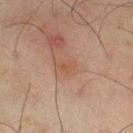Clinical impression: This lesion was catalogued during total-body skin photography and was not selected for biopsy. Context: Automated tile analysis of the lesion measured a footprint of about 3 mm², a shape eccentricity near 0.85, and a shape-asymmetry score of about 0.3 (0 = symmetric). The software also gave a lesion-to-skin contrast of about 5.5 (normalized; higher = more distinct). And it measured an automated nevus-likeness rating near 0 out of 100 and lesion-presence confidence of about 100/100. The patient is a male roughly 45 years of age. Located on the right thigh. The tile uses cross-polarized illumination. Approximately 2.5 mm at its widest. A roughly 15 mm field-of-view crop from a total-body skin photograph.